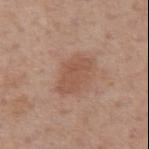Imaged during a routine full-body skin examination; the lesion was not biopsied and no histopathology is available.
A 15 mm close-up tile from a total-body photography series done for melanoma screening.
The lesion's longest dimension is about 4.5 mm.
On the arm.
Captured under white-light illumination.
The subject is a male approximately 60 years of age.
Automated tile analysis of the lesion measured border irregularity of about 3 on a 0–10 scale, a within-lesion color-variation index near 2/10, and radial color variation of about 0.5.